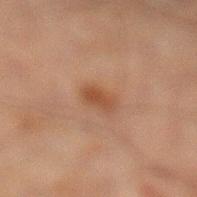Approximately 2.5 mm at its widest.
Cropped from a whole-body photographic skin survey; the tile spans about 15 mm.
A male patient aged 58 to 62.
Automated image analysis of the tile measured a lesion area of about 3.5 mm², a shape eccentricity near 0.8, and a symmetry-axis asymmetry near 0.25. The software also gave a mean CIELAB color near L≈36 a*≈18 b*≈27 and roughly 7 lightness units darker than nearby skin. The analysis additionally found border irregularity of about 2.5 on a 0–10 scale, internal color variation of about 1.5 on a 0–10 scale, and radial color variation of about 0.5.
From the left lower leg.